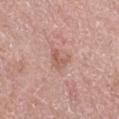Q: Was this lesion biopsied?
A: total-body-photography surveillance lesion; no biopsy
Q: What is the lesion's diameter?
A: about 2.5 mm
Q: What are the patient's age and sex?
A: female, about 65 years old
Q: What did automated image analysis measure?
A: an average lesion color of about L≈58 a*≈22 b*≈27 (CIELAB) and about 8 CIELAB-L* units darker than the surrounding skin
Q: What is the anatomic site?
A: the right thigh
Q: What kind of image is this?
A: ~15 mm tile from a whole-body skin photo
Q: Illumination type?
A: white-light illumination